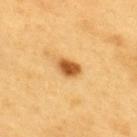{
  "biopsy_status": "not biopsied; imaged during a skin examination",
  "automated_metrics": {
    "cielab_L": 57,
    "cielab_a": 25,
    "cielab_b": 47,
    "vs_skin_darker_L": 17.0,
    "vs_skin_contrast_norm": 11.0,
    "nevus_likeness_0_100": 100,
    "lesion_detection_confidence_0_100": 100
  },
  "lighting": "cross-polarized",
  "image": {
    "source": "total-body photography crop",
    "field_of_view_mm": 15
  },
  "site": "upper back",
  "patient": {
    "sex": "male",
    "age_approx": 60
  },
  "lesion_size": {
    "long_diameter_mm_approx": 3.0
  }
}